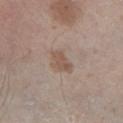Captured during whole-body skin photography for melanoma surveillance; the lesion was not biopsied. The lesion-visualizer software estimated an average lesion color of about L≈53 a*≈15 b*≈26 (CIELAB), a lesion–skin lightness drop of about 8, and a normalized lesion–skin contrast near 6.5. The analysis additionally found a border-irregularity index near 4/10, a within-lesion color-variation index near 2.5/10, and radial color variation of about 1. The software also gave a classifier nevus-likeness of about 5/100. A lesion tile, about 15 mm wide, cut from a 3D total-body photograph. Approximately 3.5 mm at its widest. A male subject in their 60s. This is a white-light tile. Located on the right lower leg.